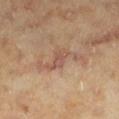Impression:
Imaged during a routine full-body skin examination; the lesion was not biopsied and no histopathology is available.
Acquisition and patient details:
The lesion's longest dimension is about 6.5 mm. The lesion is on the left lower leg. A female patient, aged 58–62. A 15 mm close-up extracted from a 3D total-body photography capture. Automated tile analysis of the lesion measured a shape eccentricity near 0.9 and two-axis asymmetry of about 0.7. The software also gave a mean CIELAB color near L≈55 a*≈20 b*≈29 and a lesion–skin lightness drop of about 8. It also reported an automated nevus-likeness rating near 0 out of 100. This is a cross-polarized tile.